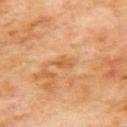<case>
<biopsy_status>not biopsied; imaged during a skin examination</biopsy_status>
<image>
  <source>total-body photography crop</source>
  <field_of_view_mm>15</field_of_view_mm>
</image>
<site>mid back</site>
<lesion_size>
  <long_diameter_mm_approx>3.0</long_diameter_mm_approx>
</lesion_size>
<lighting>cross-polarized</lighting>
<patient>
  <sex>male</sex>
  <age_approx>70</age_approx>
</patient>
</case>